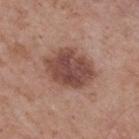Impression:
The lesion was photographed on a routine skin check and not biopsied; there is no pathology result.
Clinical summary:
A 15 mm crop from a total-body photograph taken for skin-cancer surveillance. The recorded lesion diameter is about 6 mm. From the upper back. A male patient roughly 70 years of age. The total-body-photography lesion software estimated about 13 CIELAB-L* units darker than the surrounding skin. The analysis additionally found a border-irregularity index near 1.5/10, a within-lesion color-variation index near 4/10, and radial color variation of about 1.5. The analysis additionally found a classifier nevus-likeness of about 55/100 and a lesion-detection confidence of about 100/100. The tile uses white-light illumination.